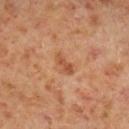Q: Was this lesion biopsied?
A: total-body-photography surveillance lesion; no biopsy
Q: Patient demographics?
A: male, about 60 years old
Q: How was this image acquired?
A: 15 mm crop, total-body photography
Q: How was the tile lit?
A: cross-polarized illumination
Q: Lesion location?
A: the leg
Q: Lesion size?
A: ≈3 mm